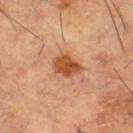patient: male, roughly 65 years of age
image source: ~15 mm tile from a whole-body skin photo
diameter: ~3.5 mm (longest diameter)
anatomic site: the right thigh
tile lighting: cross-polarized illumination